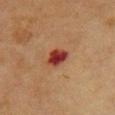anatomic site: the chest | subject: female, about 55 years old | image source: ~15 mm tile from a whole-body skin photo.